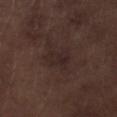workup: no biopsy performed (imaged during a skin exam) | body site: the right lower leg | acquisition: ~15 mm tile from a whole-body skin photo | subject: male, approximately 70 years of age | lighting: white-light illumination.